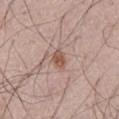This lesion was catalogued during total-body skin photography and was not selected for biopsy. From the front of the torso. Measured at roughly 2.5 mm in maximum diameter. A male patient, in their mid-60s. The lesion-visualizer software estimated a footprint of about 3.5 mm², an eccentricity of roughly 0.75, and a shape-asymmetry score of about 0.25 (0 = symmetric). The software also gave roughly 10 lightness units darker than nearby skin and a normalized lesion–skin contrast near 8. The software also gave border irregularity of about 2.5 on a 0–10 scale. And it measured a nevus-likeness score of about 80/100 and a lesion-detection confidence of about 100/100. Cropped from a total-body skin-imaging series; the visible field is about 15 mm. The tile uses white-light illumination.